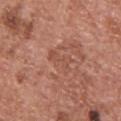Assessment:
This lesion was catalogued during total-body skin photography and was not selected for biopsy.
Clinical summary:
The lesion-visualizer software estimated a shape eccentricity near 0.9 and a symmetry-axis asymmetry near 0.5. The software also gave a mean CIELAB color near L≈51 a*≈24 b*≈29, roughly 6 lightness units darker than nearby skin, and a normalized border contrast of about 4.5. It also reported border irregularity of about 7 on a 0–10 scale and a peripheral color-asymmetry measure near 0. The analysis additionally found a classifier nevus-likeness of about 0/100 and lesion-presence confidence of about 100/100. Measured at roughly 4 mm in maximum diameter. A male patient aged 68 to 72. From the back. A close-up tile cropped from a whole-body skin photograph, about 15 mm across.